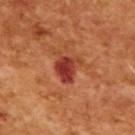Assessment:
Captured during whole-body skin photography for melanoma surveillance; the lesion was not biopsied.
Context:
The subject is a male in their mid-60s. The tile uses cross-polarized illumination. The lesion's longest dimension is about 3.5 mm. A region of skin cropped from a whole-body photographic capture, roughly 15 mm wide.